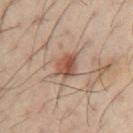This lesion was catalogued during total-body skin photography and was not selected for biopsy. From the arm. A male subject roughly 40 years of age. A region of skin cropped from a whole-body photographic capture, roughly 15 mm wide.